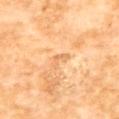The tile uses cross-polarized illumination. About 3 mm across. An algorithmic analysis of the crop reported a border-irregularity index near 7/10 and internal color variation of about 0 on a 0–10 scale. The analysis additionally found a nevus-likeness score of about 0/100 and a lesion-detection confidence of about 55/100. A female subject, about 55 years old. A region of skin cropped from a whole-body photographic capture, roughly 15 mm wide. Located on the upper back.